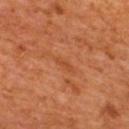image — 15 mm crop, total-body photography
location — the upper back
patient — male, in their mid- to late 60s
lesion diameter — about 3 mm